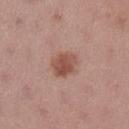Clinical impression:
Captured during whole-body skin photography for melanoma surveillance; the lesion was not biopsied.
Context:
The lesion is on the left lower leg. Approximately 3.5 mm at its widest. A 15 mm crop from a total-body photograph taken for skin-cancer surveillance. Captured under white-light illumination. A female subject roughly 55 years of age.